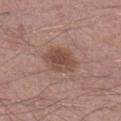| feature | finding |
|---|---|
| follow-up | imaged on a skin check; not biopsied |
| anatomic site | the right lower leg |
| subject | male, about 55 years old |
| tile lighting | white-light illumination |
| acquisition | ~15 mm tile from a whole-body skin photo |
| TBP lesion metrics | two-axis asymmetry of about 0.2; a lesion color around L≈48 a*≈20 b*≈25 in CIELAB, a lesion–skin lightness drop of about 10, and a normalized lesion–skin contrast near 7.5 |